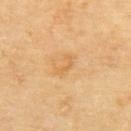{
  "biopsy_status": "not biopsied; imaged during a skin examination",
  "lighting": "cross-polarized",
  "site": "back",
  "automated_metrics": {
    "border_irregularity_0_10": 6.0,
    "color_variation_0_10": 0.0,
    "peripheral_color_asymmetry": 0.0
  },
  "image": {
    "source": "total-body photography crop",
    "field_of_view_mm": 15
  },
  "patient": {
    "sex": "female",
    "age_approx": 70
  }
}